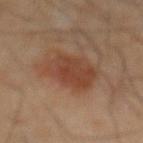Context: Measured at roughly 5 mm in maximum diameter. A 15 mm close-up tile from a total-body photography series done for melanoma screening. Captured under cross-polarized illumination. Located on the front of the torso. A male patient aged 63 to 67.